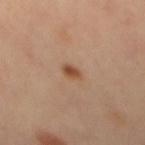Q: Was this lesion biopsied?
A: no biopsy performed (imaged during a skin exam)
Q: Patient demographics?
A: female, aged 33 to 37
Q: What is the imaging modality?
A: 15 mm crop, total-body photography
Q: What lighting was used for the tile?
A: cross-polarized
Q: What is the lesion's diameter?
A: ≈2.5 mm
Q: Where on the body is the lesion?
A: the mid back